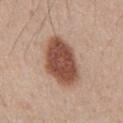Clinical summary: A close-up tile cropped from a whole-body skin photograph, about 15 mm across. A male subject aged around 60. Located on the abdomen. The total-body-photography lesion software estimated an area of roughly 21 mm². It also reported a border-irregularity index near 1.5/10, internal color variation of about 4 on a 0–10 scale, and peripheral color asymmetry of about 1. It also reported lesion-presence confidence of about 100/100. The tile uses white-light illumination. Longest diameter approximately 6.5 mm.